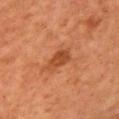The lesion was tiled from a total-body skin photograph and was not biopsied. The subject is a female aged approximately 60. This is a cross-polarized tile. From the chest. Longest diameter approximately 3.5 mm. A 15 mm crop from a total-body photograph taken for skin-cancer surveillance.